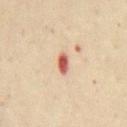The lesion was photographed on a routine skin check and not biopsied; there is no pathology result.